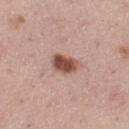Imaged during a routine full-body skin examination; the lesion was not biopsied and no histopathology is available. This image is a 15 mm lesion crop taken from a total-body photograph. An algorithmic analysis of the crop reported an area of roughly 6 mm², a shape eccentricity near 0.7, and a symmetry-axis asymmetry near 0.25. The analysis additionally found a mean CIELAB color near L≈50 a*≈22 b*≈26 and a normalized lesion–skin contrast near 11. And it measured internal color variation of about 4 on a 0–10 scale. And it measured an automated nevus-likeness rating near 95 out of 100 and a lesion-detection confidence of about 100/100. Captured under white-light illumination. Approximately 3 mm at its widest. Located on the left thigh. The patient is a female roughly 40 years of age.